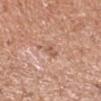Recorded during total-body skin imaging; not selected for excision or biopsy. A male subject, approximately 55 years of age. A 15 mm close-up extracted from a 3D total-body photography capture. The lesion is located on the right forearm. Measured at roughly 3 mm in maximum diameter.